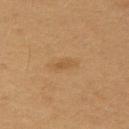The lesion was photographed on a routine skin check and not biopsied; there is no pathology result. Cropped from a whole-body photographic skin survey; the tile spans about 15 mm. Captured under cross-polarized illumination. Measured at roughly 2.5 mm in maximum diameter. On the chest. A male patient approximately 55 years of age.